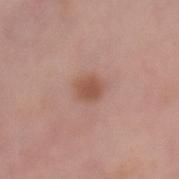Case summary:
– workup — catalogued during a skin exam; not biopsied
– patient — female, aged approximately 65
– lighting — white-light
– automated metrics — an area of roughly 4.5 mm², an eccentricity of roughly 0.5, and a symmetry-axis asymmetry near 0.15; about 10 CIELAB-L* units darker than the surrounding skin; an automated nevus-likeness rating near 90 out of 100
– location — the chest
– diameter — ~2.5 mm (longest diameter)
– image — ~15 mm tile from a whole-body skin photo